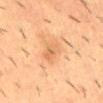follow-up=no biopsy performed (imaged during a skin exam)
subject=male, roughly 55 years of age
body site=the mid back
acquisition=~15 mm crop, total-body skin-cancer survey